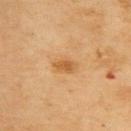Q: Was this lesion biopsied?
A: total-body-photography surveillance lesion; no biopsy
Q: Who is the patient?
A: female, in their mid-50s
Q: Lesion location?
A: the upper back
Q: Illumination type?
A: cross-polarized illumination
Q: How large is the lesion?
A: ~3 mm (longest diameter)
Q: What did automated image analysis measure?
A: a lesion area of about 4.5 mm², an outline eccentricity of about 0.8 (0 = round, 1 = elongated), and a shape-asymmetry score of about 0.25 (0 = symmetric); a mean CIELAB color near L≈51 a*≈19 b*≈38 and a normalized border contrast of about 6.5; a classifier nevus-likeness of about 45/100 and a lesion-detection confidence of about 100/100
Q: What kind of image is this?
A: 15 mm crop, total-body photography